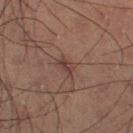Assessment:
Part of a total-body skin-imaging series; this lesion was reviewed on a skin check and was not flagged for biopsy.
Image and clinical context:
Measured at roughly 2.5 mm in maximum diameter. The lesion-visualizer software estimated an area of roughly 2.5 mm². It also reported radial color variation of about 0. Cropped from a total-body skin-imaging series; the visible field is about 15 mm. A male patient aged 58 to 62. Imaged with cross-polarized lighting.